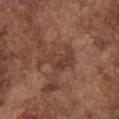follow-up = total-body-photography surveillance lesion; no biopsy
image-analysis metrics = an average lesion color of about L≈39 a*≈21 b*≈26 (CIELAB), about 7 CIELAB-L* units darker than the surrounding skin, and a normalized border contrast of about 6; a border-irregularity index near 7/10 and radial color variation of about 1.5
site = the front of the torso
patient = male, in their mid-70s
image source = ~15 mm tile from a whole-body skin photo